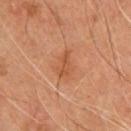| field | value |
|---|---|
| biopsy status | no biopsy performed (imaged during a skin exam) |
| tile lighting | cross-polarized illumination |
| subject | male, roughly 55 years of age |
| automated lesion analysis | a shape eccentricity near 0.85 and two-axis asymmetry of about 0.4; peripheral color asymmetry of about 0.5 |
| image | total-body-photography crop, ~15 mm field of view |
| lesion size | ≈3 mm |
| site | the chest |